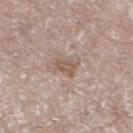Assessment: The lesion was photographed on a routine skin check and not biopsied; there is no pathology result. Acquisition and patient details: A male patient, aged approximately 75. Approximately 3 mm at its widest. A 15 mm close-up extracted from a 3D total-body photography capture. Captured under white-light illumination. The lesion is located on the left lower leg. Automated tile analysis of the lesion measured a lesion area of about 4 mm², an outline eccentricity of about 0.75 (0 = round, 1 = elongated), and a shape-asymmetry score of about 0.3 (0 = symmetric). The analysis additionally found a peripheral color-asymmetry measure near 0.5.